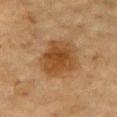Q: Was a biopsy performed?
A: imaged on a skin check; not biopsied
Q: What is the imaging modality?
A: ~15 mm tile from a whole-body skin photo
Q: What is the anatomic site?
A: the chest
Q: How was the tile lit?
A: cross-polarized
Q: What are the patient's age and sex?
A: male, in their mid- to late 80s or older